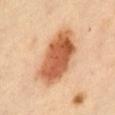  image:
    source: total-body photography crop
    field_of_view_mm: 15
  patient:
    sex: female
    age_approx: 60
  lesion_size:
    long_diameter_mm_approx: 7.5
  site: abdomen
  automated_metrics:
    cielab_L: 61
    cielab_a: 26
    cielab_b: 39
    vs_skin_darker_L: 17.0
    vs_skin_contrast_norm: 10.5
    border_irregularity_0_10: 2.0
    color_variation_0_10: 6.5
    peripheral_color_asymmetry: 2.0